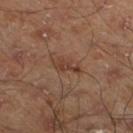Assessment: Part of a total-body skin-imaging series; this lesion was reviewed on a skin check and was not flagged for biopsy. Clinical summary: A lesion tile, about 15 mm wide, cut from a 3D total-body photograph. On the left lower leg. An algorithmic analysis of the crop reported a footprint of about 3.5 mm², a shape eccentricity near 0.9, and a shape-asymmetry score of about 0.4 (0 = symmetric). About 3 mm across. Imaged with cross-polarized lighting. A male subject, roughly 45 years of age.